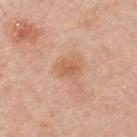Clinical impression:
Recorded during total-body skin imaging; not selected for excision or biopsy.
Acquisition and patient details:
Automated tile analysis of the lesion measured a lesion color around L≈60 a*≈24 b*≈34 in CIELAB and a lesion–skin lightness drop of about 8. The analysis additionally found border irregularity of about 2.5 on a 0–10 scale, internal color variation of about 2 on a 0–10 scale, and a peripheral color-asymmetry measure near 0.5. And it measured a nevus-likeness score of about 10/100. A lesion tile, about 15 mm wide, cut from a 3D total-body photograph. Located on the upper back. Approximately 2.5 mm at its widest. The patient is a male roughly 45 years of age.